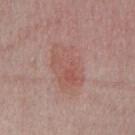Q: How was the tile lit?
A: white-light
Q: What are the patient's age and sex?
A: male, aged 53–57
Q: What is the lesion's diameter?
A: ≈6.5 mm
Q: What is the anatomic site?
A: the front of the torso
Q: How was this image acquired?
A: 15 mm crop, total-body photography